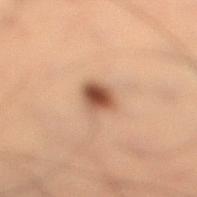Captured during whole-body skin photography for melanoma surveillance; the lesion was not biopsied. A male subject, aged approximately 55. This image is a 15 mm lesion crop taken from a total-body photograph. Located on the leg.